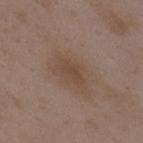Notes:
• follow-up — catalogued during a skin exam; not biopsied
• acquisition — 15 mm crop, total-body photography
• site — the upper back
• subject — female, in their mid- to late 30s
• illumination — white-light illumination